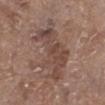{
  "biopsy_status": "not biopsied; imaged during a skin examination",
  "site": "left lower leg",
  "lighting": "white-light",
  "automated_metrics": {
    "area_mm2_approx": 24.0,
    "eccentricity": 0.85,
    "cielab_L": 46,
    "cielab_a": 17,
    "cielab_b": 23,
    "vs_skin_darker_L": 8.0,
    "vs_skin_contrast_norm": 6.5
  },
  "image": {
    "source": "total-body photography crop",
    "field_of_view_mm": 15
  },
  "patient": {
    "sex": "male",
    "age_approx": 65
  },
  "lesion_size": {
    "long_diameter_mm_approx": 7.5
  }
}